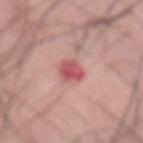Part of a total-body skin-imaging series; this lesion was reviewed on a skin check and was not flagged for biopsy.
Located on the abdomen.
The recorded lesion diameter is about 3 mm.
Imaged with white-light lighting.
A close-up tile cropped from a whole-body skin photograph, about 15 mm across.
The subject is a male aged 58 to 62.
Automated image analysis of the tile measured a lesion area of about 5.5 mm² and an outline eccentricity of about 0.65 (0 = round, 1 = elongated). The software also gave a nevus-likeness score of about 5/100 and a lesion-detection confidence of about 100/100.